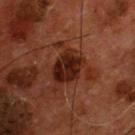Case summary:
- notes — imaged on a skin check; not biopsied